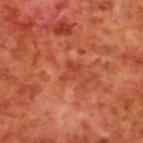* follow-up · catalogued during a skin exam; not biopsied
* tile lighting · cross-polarized
* lesion size · ≈2.5 mm
* location · the upper back
* image source · ~15 mm crop, total-body skin-cancer survey
* subject · male, roughly 70 years of age